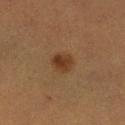Case summary:
– notes — catalogued during a skin exam; not biopsied
– subject — female, approximately 55 years of age
– imaging modality — ~15 mm crop, total-body skin-cancer survey
– TBP lesion metrics — radial color variation of about 1; an automated nevus-likeness rating near 95 out of 100 and lesion-presence confidence of about 100/100
– illumination — cross-polarized illumination
– anatomic site — the leg
– lesion size — ≈3 mm